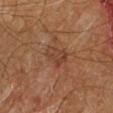Clinical impression:
Imaged during a routine full-body skin examination; the lesion was not biopsied and no histopathology is available.
Acquisition and patient details:
The total-body-photography lesion software estimated an eccentricity of roughly 0.8 and two-axis asymmetry of about 0.3. It also reported a lesion color around L≈39 a*≈21 b*≈29 in CIELAB, roughly 7 lightness units darker than nearby skin, and a normalized lesion–skin contrast near 6. The software also gave a border-irregularity rating of about 3.5/10, internal color variation of about 3.5 on a 0–10 scale, and peripheral color asymmetry of about 1.5. The lesion's longest dimension is about 3 mm. A male patient, aged approximately 60. On the left lower leg. The tile uses cross-polarized illumination. A lesion tile, about 15 mm wide, cut from a 3D total-body photograph.